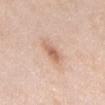Clinical impression: The lesion was photographed on a routine skin check and not biopsied; there is no pathology result. Context: On the abdomen. A 15 mm close-up extracted from a 3D total-body photography capture. A male patient in their mid-60s.